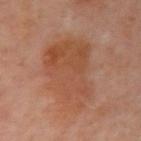automated_metrics:
  area_mm2_approx: 38.0
  eccentricity: 0.75
  shape_asymmetry: 0.2
  vs_skin_contrast_norm: 6.0
  nevus_likeness_0_100: 0
  lesion_detection_confidence_0_100: 100
site: left upper arm
patient:
  sex: female
  age_approx: 50
image:
  source: total-body photography crop
  field_of_view_mm: 15
lighting: cross-polarized